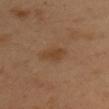<record>
<biopsy_status>not biopsied; imaged during a skin examination</biopsy_status>
<lighting>cross-polarized</lighting>
<lesion_size>
  <long_diameter_mm_approx>3.0</long_diameter_mm_approx>
</lesion_size>
<image>
  <source>total-body photography crop</source>
  <field_of_view_mm>15</field_of_view_mm>
</image>
<patient>
  <sex>male</sex>
  <age_approx>50</age_approx>
</patient>
<automated_metrics>
  <area_mm2_approx>4.0</area_mm2_approx>
  <eccentricity>0.75</eccentricity>
  <shape_asymmetry>0.3</shape_asymmetry>
  <cielab_L>41</cielab_L>
  <cielab_a>19</cielab_a>
  <cielab_b>32</cielab_b>
  <vs_skin_darker_L>6.0</vs_skin_darker_L>
  <vs_skin_contrast_norm>6.5</vs_skin_contrast_norm>
  <border_irregularity_0_10>3.0</border_irregularity_0_10>
  <color_variation_0_10>1.0</color_variation_0_10>
  <peripheral_color_asymmetry>0.5</peripheral_color_asymmetry>
</automated_metrics>
<site>arm</site>
</record>